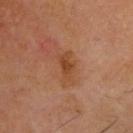location: the head or neck | acquisition: 15 mm crop, total-body photography | diameter: about 4 mm | subject: male, aged approximately 70 | illumination: cross-polarized.